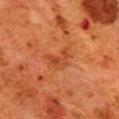The lesion-visualizer software estimated an area of roughly 6 mm², an eccentricity of roughly 0.8, and a symmetry-axis asymmetry near 0.5. The software also gave a lesion color around L≈39 a*≈27 b*≈36 in CIELAB, about 6 CIELAB-L* units darker than the surrounding skin, and a lesion-to-skin contrast of about 5 (normalized; higher = more distinct). It also reported border irregularity of about 5.5 on a 0–10 scale, internal color variation of about 3.5 on a 0–10 scale, and radial color variation of about 1.
A female subject, aged approximately 50.
On the upper back.
The lesion's longest dimension is about 4 mm.
A region of skin cropped from a whole-body photographic capture, roughly 15 mm wide.
This is a cross-polarized tile.